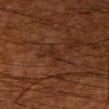workup — catalogued during a skin exam; not biopsied
anatomic site — the right upper arm
tile lighting — cross-polarized
lesion size — ~3 mm (longest diameter)
image-analysis metrics — an average lesion color of about L≈21 a*≈18 b*≈24 (CIELAB), a lesion–skin lightness drop of about 4, and a normalized lesion–skin contrast near 5; a classifier nevus-likeness of about 0/100 and lesion-presence confidence of about 60/100
acquisition — ~15 mm tile from a whole-body skin photo
subject — male, aged 58–62